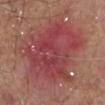Located on the right lower leg.
The recorded lesion diameter is about 10 mm.
The tile uses white-light illumination.
The patient is a male about 65 years old.
Automated image analysis of the tile measured an average lesion color of about L≈44 a*≈31 b*≈22 (CIELAB), roughly 9 lightness units darker than nearby skin, and a normalized border contrast of about 7.5. And it measured border irregularity of about 4.5 on a 0–10 scale and a within-lesion color-variation index near 6.5/10. And it measured a detector confidence of about 100 out of 100 that the crop contains a lesion.
A close-up tile cropped from a whole-body skin photograph, about 15 mm across.
The biopsy diagnosis was a nodular basal cell carcinoma (malignant).